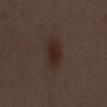The lesion was tiled from a total-body skin photograph and was not biopsied. The subject is a male aged around 50. A 15 mm crop from a total-body photograph taken for skin-cancer surveillance.